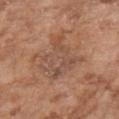Context:
A female patient aged around 75. The lesion is located on the right forearm. Approximately 5 mm at its widest. This is a white-light tile. A roughly 15 mm field-of-view crop from a total-body skin photograph. An algorithmic analysis of the crop reported an average lesion color of about L≈51 a*≈20 b*≈28 (CIELAB), a lesion–skin lightness drop of about 7, and a normalized lesion–skin contrast near 5.5. The analysis additionally found a border-irregularity rating of about 5.5/10 and a peripheral color-asymmetry measure near 1.